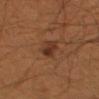* follow-up — no biopsy performed (imaged during a skin exam)
* subject — male, aged 48 to 52
* body site — the arm
* image — 15 mm crop, total-body photography